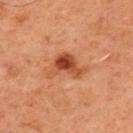Findings:
– biopsy status — total-body-photography surveillance lesion; no biopsy
– size — ≈4.5 mm
– illumination — cross-polarized illumination
– subject — male, aged 58 to 62
– image source — ~15 mm crop, total-body skin-cancer survey
– anatomic site — the left upper arm
– automated lesion analysis — a border-irregularity rating of about 6/10; a classifier nevus-likeness of about 80/100 and a detector confidence of about 100 out of 100 that the crop contains a lesion